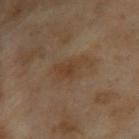biopsy status: catalogued during a skin exam; not biopsied | diameter: ≈5 mm | anatomic site: the upper back | patient: female, approximately 60 years of age | automated metrics: a lesion area of about 9.5 mm², an eccentricity of roughly 0.85, and a shape-asymmetry score of about 0.3 (0 = symmetric); a lesion color around L≈34 a*≈13 b*≈26 in CIELAB, about 5 CIELAB-L* units darker than the surrounding skin, and a normalized lesion–skin contrast near 6; a border-irregularity rating of about 4/10, internal color variation of about 2.5 on a 0–10 scale, and peripheral color asymmetry of about 1 | image: total-body-photography crop, ~15 mm field of view.